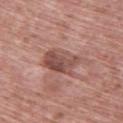This lesion was catalogued during total-body skin photography and was not selected for biopsy. The lesion is on the back. About 4.5 mm across. The patient is a male aged approximately 60. Automated image analysis of the tile measured a mean CIELAB color near L≈50 a*≈22 b*≈24, roughly 11 lightness units darker than nearby skin, and a lesion-to-skin contrast of about 8 (normalized; higher = more distinct). The software also gave an automated nevus-likeness rating near 0 out of 100 and a detector confidence of about 100 out of 100 that the crop contains a lesion. The tile uses white-light illumination. A close-up tile cropped from a whole-body skin photograph, about 15 mm across.